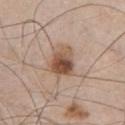Part of a total-body skin-imaging series; this lesion was reviewed on a skin check and was not flagged for biopsy.
Cropped from a total-body skin-imaging series; the visible field is about 15 mm.
The patient is a male in their mid- to late 50s.
On the front of the torso.